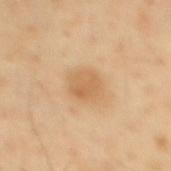{"site": "mid back", "image": {"source": "total-body photography crop", "field_of_view_mm": 15}, "lesion_size": {"long_diameter_mm_approx": 3.0}, "patient": {"sex": "male", "age_approx": 40}, "automated_metrics": {"area_mm2_approx": 6.5, "eccentricity": 0.4, "shape_asymmetry": 0.15, "border_irregularity_0_10": 1.5, "color_variation_0_10": 2.5, "peripheral_color_asymmetry": 1.0, "lesion_detection_confidence_0_100": 100}}